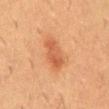Impression:
Part of a total-body skin-imaging series; this lesion was reviewed on a skin check and was not flagged for biopsy.
Background:
A male patient in their mid- to late 50s. Automated image analysis of the tile measured a mean CIELAB color near L≈51 a*≈25 b*≈35 and about 9 CIELAB-L* units darker than the surrounding skin. It also reported a classifier nevus-likeness of about 95/100 and lesion-presence confidence of about 100/100. A 15 mm crop from a total-body photograph taken for skin-cancer surveillance. Approximately 4.5 mm at its widest. The lesion is located on the mid back.